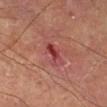The lesion was photographed on a routine skin check and not biopsied; there is no pathology result. Captured under cross-polarized illumination. Approximately 3 mm at its widest. A lesion tile, about 15 mm wide, cut from a 3D total-body photograph. The subject is a male aged around 55. On the right lower leg.